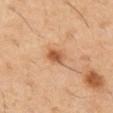Imaged during a routine full-body skin examination; the lesion was not biopsied and no histopathology is available.
Approximately 2.5 mm at its widest.
The subject is a male approximately 60 years of age.
Cropped from a whole-body photographic skin survey; the tile spans about 15 mm.
On the left thigh.
This is a cross-polarized tile.
Automated image analysis of the tile measured a lesion area of about 4.5 mm² and a shape-asymmetry score of about 0.2 (0 = symmetric). It also reported a border-irregularity index near 1.5/10. And it measured a classifier nevus-likeness of about 90/100.